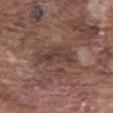This lesion was catalogued during total-body skin photography and was not selected for biopsy. This is a white-light tile. Located on the abdomen. A male subject, in their mid- to late 70s. A 15 mm close-up extracted from a 3D total-body photography capture. The lesion's longest dimension is about 5.5 mm. An algorithmic analysis of the crop reported a footprint of about 10 mm² and two-axis asymmetry of about 0.3. It also reported a lesion color around L≈40 a*≈17 b*≈22 in CIELAB, a lesion–skin lightness drop of about 7, and a lesion-to-skin contrast of about 6.5 (normalized; higher = more distinct).